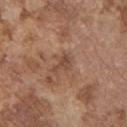biopsy status = catalogued during a skin exam; not biopsied
image = ~15 mm crop, total-body skin-cancer survey
lighting = white-light illumination
subject = male, aged approximately 75
diameter = ≈2.5 mm
anatomic site = the chest
automated metrics = roughly 8 lightness units darker than nearby skin and a normalized border contrast of about 6.5; an automated nevus-likeness rating near 0 out of 100 and lesion-presence confidence of about 100/100